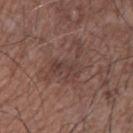| feature | finding |
|---|---|
| notes | imaged on a skin check; not biopsied |
| anatomic site | the front of the torso |
| lesion size | ≈6 mm |
| lighting | white-light illumination |
| image | total-body-photography crop, ~15 mm field of view |
| subject | male, aged approximately 70 |